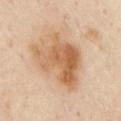Q: Was a biopsy performed?
A: catalogued during a skin exam; not biopsied
Q: What did automated image analysis measure?
A: a footprint of about 25 mm², an eccentricity of roughly 0.7, and a shape-asymmetry score of about 0.4 (0 = symmetric); a detector confidence of about 100 out of 100 that the crop contains a lesion
Q: How was this image acquired?
A: total-body-photography crop, ~15 mm field of view
Q: Patient demographics?
A: male, aged 53–57
Q: Lesion location?
A: the chest